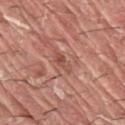image-analysis metrics: an area of roughly 3 mm², an eccentricity of roughly 0.9, and a shape-asymmetry score of about 0.35 (0 = symmetric); a lesion-to-skin contrast of about 6 (normalized; higher = more distinct); a border-irregularity index near 4.5/10, internal color variation of about 1.5 on a 0–10 scale, and radial color variation of about 0.5; a nevus-likeness score of about 0/100 and a detector confidence of about 90 out of 100 that the crop contains a lesion
site: the right upper arm
subject: male, in their 40s
lesion size: ≈3 mm
image source: 15 mm crop, total-body photography